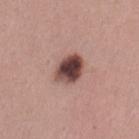Clinical summary:
The lesion is on the left thigh. The subject is a female approximately 30 years of age. Approximately 4 mm at its widest. This is a white-light tile. Cropped from a total-body skin-imaging series; the visible field is about 15 mm.